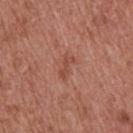This lesion was catalogued during total-body skin photography and was not selected for biopsy.
Automated tile analysis of the lesion measured a lesion area of about 3.5 mm² and two-axis asymmetry of about 0.35. The analysis additionally found a mean CIELAB color near L≈48 a*≈25 b*≈29 and roughly 7 lightness units darker than nearby skin. The analysis additionally found a border-irregularity index near 5/10, a color-variation rating of about 0/10, and peripheral color asymmetry of about 0. And it measured a classifier nevus-likeness of about 0/100.
The lesion's longest dimension is about 3 mm.
This is a white-light tile.
A male subject aged 68–72.
A lesion tile, about 15 mm wide, cut from a 3D total-body photograph.
Located on the upper back.